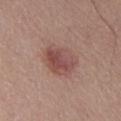Q: Is there a histopathology result?
A: no biopsy performed (imaged during a skin exam)
Q: What lighting was used for the tile?
A: white-light
Q: Lesion size?
A: about 4.5 mm
Q: Where on the body is the lesion?
A: the abdomen
Q: Automated lesion metrics?
A: a mean CIELAB color near L≈49 a*≈22 b*≈23, about 9 CIELAB-L* units darker than the surrounding skin, and a normalized lesion–skin contrast near 7; internal color variation of about 5.5 on a 0–10 scale and radial color variation of about 1.5; an automated nevus-likeness rating near 80 out of 100
Q: What kind of image is this?
A: total-body-photography crop, ~15 mm field of view
Q: Who is the patient?
A: male, about 55 years old